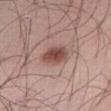Recorded during total-body skin imaging; not selected for excision or biopsy. The lesion is on the abdomen. A male patient, aged 53–57. The lesion-visualizer software estimated a mean CIELAB color near L≈49 a*≈21 b*≈23. The software also gave a nevus-likeness score of about 95/100 and a detector confidence of about 100 out of 100 that the crop contains a lesion. A lesion tile, about 15 mm wide, cut from a 3D total-body photograph.